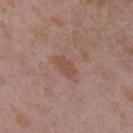| field | value |
|---|---|
| biopsy status | catalogued during a skin exam; not biopsied |
| site | the arm |
| lighting | white-light illumination |
| image source | ~15 mm tile from a whole-body skin photo |
| image-analysis metrics | a lesion area of about 3.5 mm² and an outline eccentricity of about 0.9 (0 = round, 1 = elongated); a mean CIELAB color near L≈49 a*≈19 b*≈27, about 7 CIELAB-L* units darker than the surrounding skin, and a lesion-to-skin contrast of about 6 (normalized; higher = more distinct); a border-irregularity index near 3/10, a color-variation rating of about 1/10, and peripheral color asymmetry of about 0.5 |
| subject | female, roughly 35 years of age |